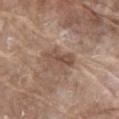Impression:
Imaged during a routine full-body skin examination; the lesion was not biopsied and no histopathology is available.
Background:
An algorithmic analysis of the crop reported a border-irregularity rating of about 4/10. A male subject, aged 78–82. Located on the abdomen. A 15 mm close-up tile from a total-body photography series done for melanoma screening. Imaged with white-light lighting. Longest diameter approximately 4 mm.